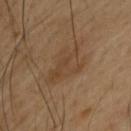Part of a total-body skin-imaging series; this lesion was reviewed on a skin check and was not flagged for biopsy. The patient is a male aged 43 to 47. On the upper back. Captured under cross-polarized illumination. A 15 mm close-up tile from a total-body photography series done for melanoma screening. The recorded lesion diameter is about 5.5 mm. The lesion-visualizer software estimated a border-irregularity index near 6/10 and a color-variation rating of about 2.5/10.